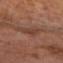The lesion was tiled from a total-body skin photograph and was not biopsied.
Automated image analysis of the tile measured a border-irregularity rating of about 4.5/10, a within-lesion color-variation index near 3.5/10, and a peripheral color-asymmetry measure near 1.5. The analysis additionally found a nevus-likeness score of about 0/100 and a lesion-detection confidence of about 95/100.
This is a cross-polarized tile.
A lesion tile, about 15 mm wide, cut from a 3D total-body photograph.
A male patient roughly 75 years of age.
On the chest.